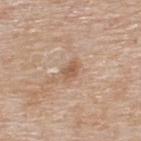biopsy status: no biopsy performed (imaged during a skin exam) | image: total-body-photography crop, ~15 mm field of view | location: the upper back | patient: male, aged 53 to 57.